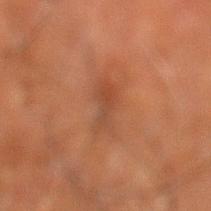Background:
The patient is a male aged around 65. A close-up tile cropped from a whole-body skin photograph, about 15 mm across. From the left lower leg. About 4 mm across. Imaged with cross-polarized lighting.